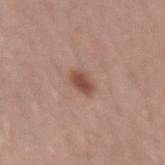{"biopsy_status": "not biopsied; imaged during a skin examination", "lighting": "white-light", "image": {"source": "total-body photography crop", "field_of_view_mm": 15}, "patient": {"sex": "male", "age_approx": 30}, "site": "left forearm"}